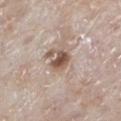No biopsy was performed on this lesion — it was imaged during a full skin examination and was not determined to be concerning. A 15 mm crop from a total-body photograph taken for skin-cancer surveillance. This is a white-light tile. Measured at roughly 2.5 mm in maximum diameter. From the left lower leg. The subject is a male in their 80s.